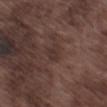A male subject, about 75 years old.
Cropped from a total-body skin-imaging series; the visible field is about 15 mm.
From the left thigh.